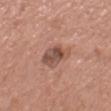workup: total-body-photography surveillance lesion; no biopsy | TBP lesion metrics: a lesion color around L≈51 a*≈21 b*≈26 in CIELAB, about 11 CIELAB-L* units darker than the surrounding skin, and a normalized border contrast of about 8; a color-variation rating of about 5.5/10; a detector confidence of about 100 out of 100 that the crop contains a lesion | tile lighting: white-light | patient: female, in their mid- to late 70s | location: the right thigh | image source: 15 mm crop, total-body photography.